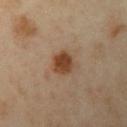Q: Was a biopsy performed?
A: imaged on a skin check; not biopsied
Q: What are the patient's age and sex?
A: female, aged approximately 40
Q: What is the imaging modality?
A: total-body-photography crop, ~15 mm field of view
Q: Lesion size?
A: about 3 mm
Q: What is the anatomic site?
A: the left upper arm
Q: What did automated image analysis measure?
A: a lesion area of about 7 mm² and an eccentricity of roughly 0.35; a border-irregularity rating of about 1.5/10, a color-variation rating of about 3.5/10, and a peripheral color-asymmetry measure near 1; a classifier nevus-likeness of about 100/100
Q: How was the tile lit?
A: cross-polarized illumination